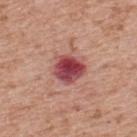Image and clinical context:
A 15 mm crop from a total-body photograph taken for skin-cancer surveillance. This is a white-light tile. The lesion is on the back. A male subject, approximately 70 years of age.